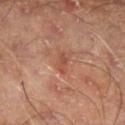Clinical impression: Part of a total-body skin-imaging series; this lesion was reviewed on a skin check and was not flagged for biopsy. Acquisition and patient details: The patient is a male aged 68–72. The lesion is on the right lower leg. This is a cross-polarized tile. A 15 mm close-up extracted from a 3D total-body photography capture.